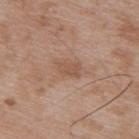• location · the upper back
• illumination · white-light
• subject · male, aged approximately 50
• lesion diameter · ~2.5 mm (longest diameter)
• acquisition · ~15 mm crop, total-body skin-cancer survey